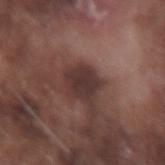Q: Is there a histopathology result?
A: total-body-photography surveillance lesion; no biopsy
Q: What are the patient's age and sex?
A: male, in their mid-70s
Q: What kind of image is this?
A: ~15 mm tile from a whole-body skin photo
Q: Lesion location?
A: the right forearm
Q: Lesion size?
A: ≈3 mm
Q: How was the tile lit?
A: white-light illumination